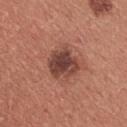Impression: The lesion was tiled from a total-body skin photograph and was not biopsied. Acquisition and patient details: This is a white-light tile. The recorded lesion diameter is about 4 mm. On the chest. A close-up tile cropped from a whole-body skin photograph, about 15 mm across. A female patient aged around 35.